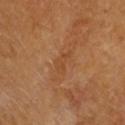follow-up: catalogued during a skin exam; not biopsied
image source: 15 mm crop, total-body photography
subject: female, aged approximately 65
site: the head or neck
automated lesion analysis: an outline eccentricity of about 0.85 (0 = round, 1 = elongated) and a shape-asymmetry score of about 0.5 (0 = symmetric); a border-irregularity rating of about 5.5/10, a color-variation rating of about 0.5/10, and a peripheral color-asymmetry measure near 0; a classifier nevus-likeness of about 0/100 and a lesion-detection confidence of about 100/100
lesion size: ≈3 mm